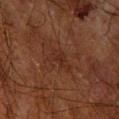Assessment: This lesion was catalogued during total-body skin photography and was not selected for biopsy. Image and clinical context: Cropped from a whole-body photographic skin survey; the tile spans about 15 mm. Captured under cross-polarized illumination. From the right forearm. Approximately 3.5 mm at its widest. The patient is a male aged around 60.